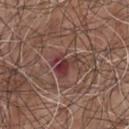{"biopsy_status": "not biopsied; imaged during a skin examination", "image": {"source": "total-body photography crop", "field_of_view_mm": 15}, "patient": {"sex": "male", "age_approx": 55}, "site": "chest", "lesion_size": {"long_diameter_mm_approx": 3.5}}